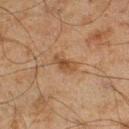No biopsy was performed on this lesion — it was imaged during a full skin examination and was not determined to be concerning. A 15 mm close-up tile from a total-body photography series done for melanoma screening. From the right lower leg. A male subject aged around 45.